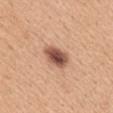No biopsy was performed on this lesion — it was imaged during a full skin examination and was not determined to be concerning. A female subject, approximately 30 years of age. The lesion is on the upper back. Automated tile analysis of the lesion measured a mean CIELAB color near L≈53 a*≈22 b*≈30, roughly 17 lightness units darker than nearby skin, and a normalized border contrast of about 11. The analysis additionally found a border-irregularity index near 2/10, internal color variation of about 6.5 on a 0–10 scale, and radial color variation of about 2. The software also gave a classifier nevus-likeness of about 90/100 and a detector confidence of about 100 out of 100 that the crop contains a lesion. A 15 mm close-up tile from a total-body photography series done for melanoma screening. The lesion's longest dimension is about 4 mm. This is a white-light tile.